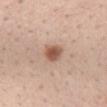This lesion was catalogued during total-body skin photography and was not selected for biopsy.
A female subject aged 33 to 37.
Captured under white-light illumination.
The lesion is located on the mid back.
Approximately 3 mm at its widest.
Automated image analysis of the tile measured a lesion area of about 5.5 mm², an outline eccentricity of about 0.65 (0 = round, 1 = elongated), and a shape-asymmetry score of about 0.2 (0 = symmetric). It also reported an average lesion color of about L≈56 a*≈20 b*≈29 (CIELAB), a lesion–skin lightness drop of about 13, and a normalized border contrast of about 9. The analysis additionally found a border-irregularity index near 2/10, a color-variation rating of about 4.5/10, and peripheral color asymmetry of about 1.5. The software also gave a classifier nevus-likeness of about 95/100 and a detector confidence of about 100 out of 100 that the crop contains a lesion.
A region of skin cropped from a whole-body photographic capture, roughly 15 mm wide.